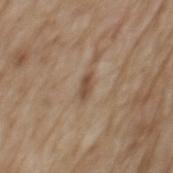Part of a total-body skin-imaging series; this lesion was reviewed on a skin check and was not flagged for biopsy. The tile uses white-light illumination. A male patient aged around 70. A lesion tile, about 15 mm wide, cut from a 3D total-body photograph. The total-body-photography lesion software estimated two-axis asymmetry of about 0.3. The software also gave a mean CIELAB color near L≈49 a*≈16 b*≈29 and roughly 10 lightness units darker than nearby skin. It also reported a border-irregularity index near 3.5/10, a within-lesion color-variation index near 0/10, and peripheral color asymmetry of about 0. It also reported a nevus-likeness score of about 30/100. The lesion is on the mid back.